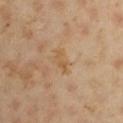Image and clinical context: Longest diameter approximately 3 mm. This is a cross-polarized tile. A male patient aged 43 to 47. Located on the right upper arm. A lesion tile, about 15 mm wide, cut from a 3D total-body photograph. The total-body-photography lesion software estimated an area of roughly 3.5 mm², an outline eccentricity of about 0.85 (0 = round, 1 = elongated), and two-axis asymmetry of about 0.5. And it measured a lesion color around L≈55 a*≈17 b*≈37 in CIELAB, about 6 CIELAB-L* units darker than the surrounding skin, and a normalized border contrast of about 6. And it measured lesion-presence confidence of about 100/100.